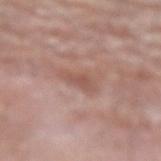The lesion was tiled from a total-body skin photograph and was not biopsied.
Captured under white-light illumination.
The lesion's longest dimension is about 3.5 mm.
The lesion is located on the arm.
The subject is a male aged around 80.
A lesion tile, about 15 mm wide, cut from a 3D total-body photograph.